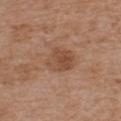Part of a total-body skin-imaging series; this lesion was reviewed on a skin check and was not flagged for biopsy.
Longest diameter approximately 4 mm.
Imaged with white-light lighting.
The patient is a male aged approximately 75.
The lesion is located on the chest.
A close-up tile cropped from a whole-body skin photograph, about 15 mm across.